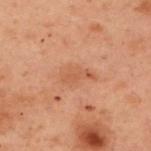Part of a total-body skin-imaging series; this lesion was reviewed on a skin check and was not flagged for biopsy. Located on the upper back. A close-up tile cropped from a whole-body skin photograph, about 15 mm across. A female subject, aged 53 to 57.